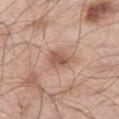The lesion was tiled from a total-body skin photograph and was not biopsied.
A male patient, roughly 70 years of age.
This is a white-light tile.
From the right thigh.
About 3.5 mm across.
A roughly 15 mm field-of-view crop from a total-body skin photograph.